A 15 mm close-up extracted from a 3D total-body photography capture. On the right upper arm. The subject is a female in their mid-40s — 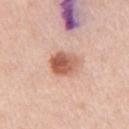| field | value |
|---|---|
| lesion diameter | ≈3.5 mm |
| image-analysis metrics | a lesion color around L≈60 a*≈25 b*≈31 in CIELAB and a lesion-to-skin contrast of about 9 (normalized; higher = more distinct); an automated nevus-likeness rating near 100 out of 100 and a detector confidence of about 100 out of 100 that the crop contains a lesion |
| tile lighting | white-light illumination |
| histopathologic diagnosis | a dysplastic (Clark) nevus |